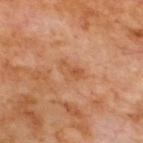follow-up=catalogued during a skin exam; not biopsied
illumination=cross-polarized illumination
automated metrics=a footprint of about 5 mm², a shape eccentricity near 0.8, and a symmetry-axis asymmetry near 0.4; border irregularity of about 4 on a 0–10 scale, internal color variation of about 3.5 on a 0–10 scale, and radial color variation of about 1
diameter=about 3.5 mm
imaging modality=total-body-photography crop, ~15 mm field of view
patient=male, aged around 70
anatomic site=the upper back